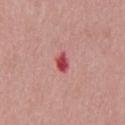Q: Was this lesion biopsied?
A: catalogued during a skin exam; not biopsied
Q: What kind of image is this?
A: ~15 mm crop, total-body skin-cancer survey
Q: Where on the body is the lesion?
A: the chest
Q: What are the patient's age and sex?
A: male, aged around 50
Q: Automated lesion metrics?
A: a footprint of about 3.5 mm², a shape eccentricity near 0.8, and a symmetry-axis asymmetry near 0.3; a lesion color around L≈50 a*≈39 b*≈23 in CIELAB, about 15 CIELAB-L* units darker than the surrounding skin, and a normalized border contrast of about 10; a border-irregularity index near 2.5/10 and radial color variation of about 0.5
Q: What lighting was used for the tile?
A: white-light illumination